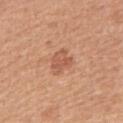| field | value |
|---|---|
| follow-up | imaged on a skin check; not biopsied |
| subject | female, aged 48 to 52 |
| lesion size | about 3 mm |
| body site | the right upper arm |
| imaging modality | total-body-photography crop, ~15 mm field of view |
| tile lighting | white-light illumination |